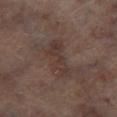Background: The tile uses cross-polarized illumination. Cropped from a whole-body photographic skin survey; the tile spans about 15 mm. From the left lower leg. The recorded lesion diameter is about 5.5 mm. A male subject aged 63 to 67.